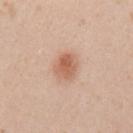follow-up: catalogued during a skin exam; not biopsied | body site: the left upper arm | image: ~15 mm tile from a whole-body skin photo | size: about 3.5 mm | subject: female, approximately 20 years of age | tile lighting: white-light.